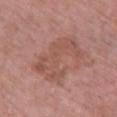workup: imaged on a skin check; not biopsied | image: ~15 mm crop, total-body skin-cancer survey | subject: female, aged 63 to 67 | anatomic site: the upper back | tile lighting: white-light illumination.